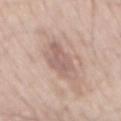Part of a total-body skin-imaging series; this lesion was reviewed on a skin check and was not flagged for biopsy.
A male subject, roughly 50 years of age.
From the mid back.
The total-body-photography lesion software estimated border irregularity of about 3 on a 0–10 scale, internal color variation of about 3.5 on a 0–10 scale, and a peripheral color-asymmetry measure near 1.5. The analysis additionally found a nevus-likeness score of about 0/100.
A 15 mm close-up extracted from a 3D total-body photography capture.
The tile uses white-light illumination.
The recorded lesion diameter is about 6 mm.